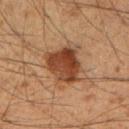The lesion was tiled from a total-body skin photograph and was not biopsied. The patient is a male aged 48–52. A close-up tile cropped from a whole-body skin photograph, about 15 mm across. Captured under cross-polarized illumination. The lesion is on the right upper arm. The recorded lesion diameter is about 5 mm.